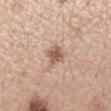image-analysis metrics = a border-irregularity rating of about 2/10, a color-variation rating of about 2/10, and a peripheral color-asymmetry measure near 0.5; subject = female, aged 43–47; lighting = white-light illumination; body site = the left forearm; diameter = ~2.5 mm (longest diameter); image source = 15 mm crop, total-body photography.